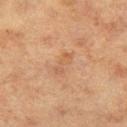A close-up tile cropped from a whole-body skin photograph, about 15 mm across.
The patient is a female roughly 40 years of age.
Approximately 3 mm at its widest.
From the left thigh.
The tile uses cross-polarized illumination.